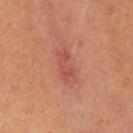| feature | finding |
|---|---|
| follow-up | imaged on a skin check; not biopsied |
| acquisition | total-body-photography crop, ~15 mm field of view |
| lighting | cross-polarized |
| size | ≈4 mm |
| image-analysis metrics | a lesion area of about 5.5 mm², an outline eccentricity of about 0.95 (0 = round, 1 = elongated), and a shape-asymmetry score of about 0.25 (0 = symmetric); a lesion color around L≈40 a*≈25 b*≈23 in CIELAB and roughly 7 lightness units darker than nearby skin; a color-variation rating of about 1/10 and radial color variation of about 0.5; a classifier nevus-likeness of about 35/100 and a lesion-detection confidence of about 100/100 |
| location | the left upper arm |
| patient | male, in their 40s |